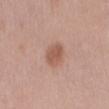workup: catalogued during a skin exam; not biopsied
body site: the front of the torso
acquisition: 15 mm crop, total-body photography
illumination: white-light illumination
patient: female, approximately 40 years of age